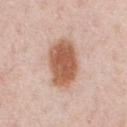Clinical impression:
Part of a total-body skin-imaging series; this lesion was reviewed on a skin check and was not flagged for biopsy.
Image and clinical context:
The subject is a male aged approximately 60. The lesion-visualizer software estimated two-axis asymmetry of about 0.15. The analysis additionally found a mean CIELAB color near L≈58 a*≈22 b*≈32 and roughly 15 lightness units darker than nearby skin. And it measured a border-irregularity index near 2/10, a within-lesion color-variation index near 4/10, and a peripheral color-asymmetry measure near 1. It also reported a lesion-detection confidence of about 100/100. Longest diameter approximately 6 mm. This is a white-light tile. The lesion is on the chest. Cropped from a total-body skin-imaging series; the visible field is about 15 mm.